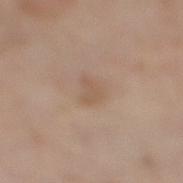biopsy status=imaged on a skin check; not biopsied | lighting=white-light | subject=female, roughly 55 years of age | body site=the right lower leg | imaging modality=total-body-photography crop, ~15 mm field of view | size=~2.5 mm (longest diameter) | image-analysis metrics=about 6 CIELAB-L* units darker than the surrounding skin; a border-irregularity index near 3.5/10 and a color-variation rating of about 1.5/10.